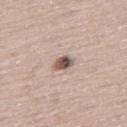Clinical impression: Recorded during total-body skin imaging; not selected for excision or biopsy. Context: Longest diameter approximately 2.5 mm. A male patient, in their mid- to late 60s. An algorithmic analysis of the crop reported a border-irregularity index near 2/10, a within-lesion color-variation index near 6.5/10, and radial color variation of about 2. The lesion is located on the right thigh. A 15 mm close-up tile from a total-body photography series done for melanoma screening. Captured under white-light illumination.